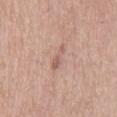Impression: Part of a total-body skin-imaging series; this lesion was reviewed on a skin check and was not flagged for biopsy. Clinical summary: A female subject aged around 60. Located on the mid back. An algorithmic analysis of the crop reported a lesion area of about 3.5 mm², an eccentricity of roughly 0.95, and a symmetry-axis asymmetry near 0.35. The analysis additionally found a mean CIELAB color near L≈59 a*≈20 b*≈26, about 8 CIELAB-L* units darker than the surrounding skin, and a lesion-to-skin contrast of about 5.5 (normalized; higher = more distinct). And it measured border irregularity of about 4.5 on a 0–10 scale and a color-variation rating of about 0.5/10. The software also gave a lesion-detection confidence of about 100/100. A 15 mm close-up extracted from a 3D total-body photography capture.